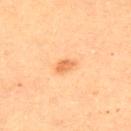Imaged during a routine full-body skin examination; the lesion was not biopsied and no histopathology is available.
A female subject, about 45 years old.
Cropped from a total-body skin-imaging series; the visible field is about 15 mm.
On the upper back.
This is a cross-polarized tile.